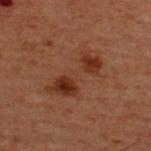Impression:
Captured during whole-body skin photography for melanoma surveillance; the lesion was not biopsied.
Background:
Located on the upper back. A male patient, about 60 years old. A 15 mm close-up tile from a total-body photography series done for melanoma screening. The tile uses cross-polarized illumination. About 7 mm across. Automated image analysis of the tile measured an automated nevus-likeness rating near 40 out of 100 and lesion-presence confidence of about 100/100.